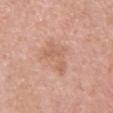  biopsy_status: not biopsied; imaged during a skin examination
  lesion_size:
    long_diameter_mm_approx: 5.0
  patient:
    sex: female
    age_approx: 30
  image:
    source: total-body photography crop
    field_of_view_mm: 15
  site: front of the torso
  lighting: white-light